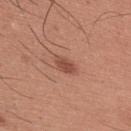Impression:
Recorded during total-body skin imaging; not selected for excision or biopsy.
Acquisition and patient details:
The subject is a male roughly 35 years of age. The recorded lesion diameter is about 3 mm. From the back. A 15 mm crop from a total-body photograph taken for skin-cancer surveillance.